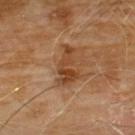The lesion was photographed on a routine skin check and not biopsied; there is no pathology result. The tile uses cross-polarized illumination. Automated image analysis of the tile measured a mean CIELAB color near L≈41 a*≈21 b*≈34, about 9 CIELAB-L* units darker than the surrounding skin, and a normalized border contrast of about 8. It also reported border irregularity of about 6 on a 0–10 scale, a within-lesion color-variation index near 4.5/10, and radial color variation of about 1.5. A 15 mm close-up extracted from a 3D total-body photography capture. The subject is a male aged 58 to 62. On the chest.